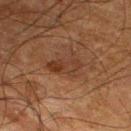workup = total-body-photography surveillance lesion; no biopsy
site = the left lower leg
image = ~15 mm crop, total-body skin-cancer survey
tile lighting = cross-polarized illumination
subject = male, aged around 65
lesion size = ≈3.5 mm